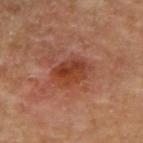Imaged during a routine full-body skin examination; the lesion was not biopsied and no histopathology is available. The lesion is on the right upper arm. A male patient in their mid- to late 50s. A close-up tile cropped from a whole-body skin photograph, about 15 mm across.